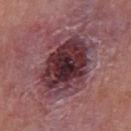Clinical impression:
The lesion was photographed on a routine skin check and not biopsied; there is no pathology result.
Context:
A male subject, aged 48 to 52. The lesion is located on the mid back. A lesion tile, about 15 mm wide, cut from a 3D total-body photograph.